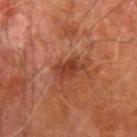The lesion was tiled from a total-body skin photograph and was not biopsied.
Longest diameter approximately 4 mm.
From the arm.
The tile uses cross-polarized illumination.
A male patient, in their 80s.
A roughly 15 mm field-of-view crop from a total-body skin photograph.
Automated tile analysis of the lesion measured an area of roughly 5 mm², an outline eccentricity of about 0.9 (0 = round, 1 = elongated), and a shape-asymmetry score of about 0.3 (0 = symmetric). And it measured an automated nevus-likeness rating near 5 out of 100.